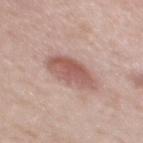| key | value |
|---|---|
| notes | no biopsy performed (imaged during a skin exam) |
| lighting | white-light illumination |
| image source | 15 mm crop, total-body photography |
| patient | female, approximately 40 years of age |
| automated metrics | an eccentricity of roughly 0.8; an automated nevus-likeness rating near 90 out of 100 and a lesion-detection confidence of about 100/100 |
| location | the upper back |